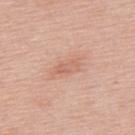Recorded during total-body skin imaging; not selected for excision or biopsy. Approximately 3.5 mm at its widest. An algorithmic analysis of the crop reported an eccentricity of roughly 0.9 and a shape-asymmetry score of about 0.3 (0 = symmetric). And it measured an average lesion color of about L≈63 a*≈24 b*≈30 (CIELAB) and roughly 7 lightness units darker than nearby skin. And it measured border irregularity of about 3.5 on a 0–10 scale, a within-lesion color-variation index near 1.5/10, and peripheral color asymmetry of about 0.5. The software also gave lesion-presence confidence of about 100/100. Located on the upper back. The patient is a male aged 53–57. A lesion tile, about 15 mm wide, cut from a 3D total-body photograph.